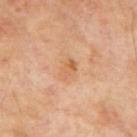Part of a total-body skin-imaging series; this lesion was reviewed on a skin check and was not flagged for biopsy.
Captured under cross-polarized illumination.
A male subject about 70 years old.
The lesion is on the mid back.
Cropped from a whole-body photographic skin survey; the tile spans about 15 mm.
Measured at roughly 3 mm in maximum diameter.
The total-body-photography lesion software estimated a mean CIELAB color near L≈61 a*≈22 b*≈38, about 7 CIELAB-L* units darker than the surrounding skin, and a normalized border contrast of about 5.5. And it measured border irregularity of about 2.5 on a 0–10 scale, a within-lesion color-variation index near 6/10, and a peripheral color-asymmetry measure near 2. It also reported an automated nevus-likeness rating near 0 out of 100 and a lesion-detection confidence of about 100/100.